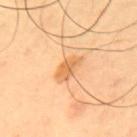Background:
A 15 mm close-up extracted from a 3D total-body photography capture. The patient is a male aged around 65. The lesion's longest dimension is about 3.5 mm. The lesion is located on the mid back. Captured under cross-polarized illumination. The total-body-photography lesion software estimated an average lesion color of about L≈68 a*≈25 b*≈45 (CIELAB), roughly 10 lightness units darker than nearby skin, and a normalized border contrast of about 7.